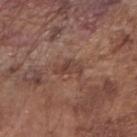The lesion was tiled from a total-body skin photograph and was not biopsied. The recorded lesion diameter is about 3.5 mm. The subject is a male in their mid-70s. A close-up tile cropped from a whole-body skin photograph, about 15 mm across. Captured under white-light illumination. The lesion-visualizer software estimated a footprint of about 4.5 mm², an eccentricity of roughly 0.85, and a shape-asymmetry score of about 0.45 (0 = symmetric). The analysis additionally found a mean CIELAB color near L≈42 a*≈20 b*≈25 and a lesion-to-skin contrast of about 6.5 (normalized; higher = more distinct). The analysis additionally found radial color variation of about 0.5. The analysis additionally found a classifier nevus-likeness of about 0/100 and a lesion-detection confidence of about 95/100. Located on the right forearm.